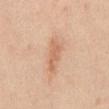body site: the front of the torso; illumination: white-light; subject: male, in their mid- to late 40s; imaging modality: ~15 mm tile from a whole-body skin photo; automated metrics: a nevus-likeness score of about 0/100.